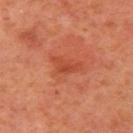Impression: Captured during whole-body skin photography for melanoma surveillance; the lesion was not biopsied. Background: The lesion-visualizer software estimated a footprint of about 4.5 mm², an eccentricity of roughly 0.85, and two-axis asymmetry of about 0.55. The software also gave a lesion color around L≈49 a*≈35 b*≈38 in CIELAB, roughly 8 lightness units darker than nearby skin, and a lesion-to-skin contrast of about 5.5 (normalized; higher = more distinct). The software also gave a border-irregularity rating of about 5.5/10, a within-lesion color-variation index near 1.5/10, and a peripheral color-asymmetry measure near 0.5. And it measured a classifier nevus-likeness of about 0/100. Located on the left upper arm. A roughly 15 mm field-of-view crop from a total-body skin photograph. A female subject about 40 years old. The tile uses cross-polarized illumination. About 3.5 mm across.